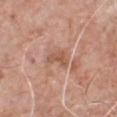The tile uses white-light illumination. The recorded lesion diameter is about 3 mm. The subject is a male aged 58 to 62. Located on the front of the torso. A roughly 15 mm field-of-view crop from a total-body skin photograph.